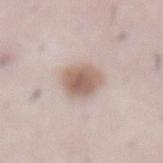Captured during whole-body skin photography for melanoma surveillance; the lesion was not biopsied. Imaged with white-light lighting. A male patient, approximately 50 years of age. Automated tile analysis of the lesion measured an area of roughly 10 mm² and an outline eccentricity of about 0.5 (0 = round, 1 = elongated). And it measured a classifier nevus-likeness of about 90/100 and a detector confidence of about 100 out of 100 that the crop contains a lesion. Cropped from a whole-body photographic skin survey; the tile spans about 15 mm. Longest diameter approximately 4 mm. On the abdomen.